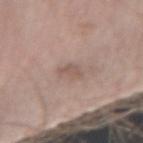The lesion was tiled from a total-body skin photograph and was not biopsied.
This is a white-light tile.
Located on the arm.
A region of skin cropped from a whole-body photographic capture, roughly 15 mm wide.
The subject is a male about 60 years old.
Measured at roughly 2.5 mm in maximum diameter.
The lesion-visualizer software estimated a border-irregularity index near 3.5/10, a color-variation rating of about 1/10, and radial color variation of about 0.5. The software also gave lesion-presence confidence of about 100/100.